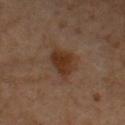Captured during whole-body skin photography for melanoma surveillance; the lesion was not biopsied.
The tile uses cross-polarized illumination.
The lesion is located on the front of the torso.
The lesion's longest dimension is about 4 mm.
A male subject, approximately 50 years of age.
An algorithmic analysis of the crop reported a lesion color around L≈32 a*≈19 b*≈28 in CIELAB, a lesion–skin lightness drop of about 9, and a lesion-to-skin contrast of about 9 (normalized; higher = more distinct).
A lesion tile, about 15 mm wide, cut from a 3D total-body photograph.